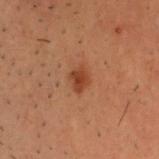Case summary:
* workup: total-body-photography surveillance lesion; no biopsy
* site: the head or neck
* image: 15 mm crop, total-body photography
* illumination: cross-polarized illumination
* patient: male, about 30 years old
* automated lesion analysis: a shape eccentricity near 0.7 and a symmetry-axis asymmetry near 0.15; a lesion color around L≈34 a*≈22 b*≈29 in CIELAB; a border-irregularity index near 1.5/10, a color-variation rating of about 2/10, and a peripheral color-asymmetry measure near 0.5